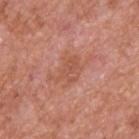Clinical impression: No biopsy was performed on this lesion — it was imaged during a full skin examination and was not determined to be concerning. Image and clinical context: Imaged with white-light lighting. The recorded lesion diameter is about 3.5 mm. From the upper back. A region of skin cropped from a whole-body photographic capture, roughly 15 mm wide. A male patient aged approximately 65.